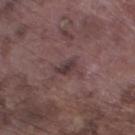No biopsy was performed on this lesion — it was imaged during a full skin examination and was not determined to be concerning.
The tile uses white-light illumination.
A male subject, aged approximately 75.
From the left lower leg.
The lesion-visualizer software estimated a footprint of about 3.5 mm², an outline eccentricity of about 0.75 (0 = round, 1 = elongated), and two-axis asymmetry of about 0.4. The analysis additionally found a lesion-to-skin contrast of about 7.5 (normalized; higher = more distinct). And it measured a nevus-likeness score of about 0/100 and a lesion-detection confidence of about 95/100.
A region of skin cropped from a whole-body photographic capture, roughly 15 mm wide.
Longest diameter approximately 3 mm.